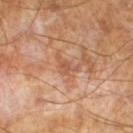{
  "biopsy_status": "not biopsied; imaged during a skin examination",
  "patient": {
    "sex": "male",
    "age_approx": 65
  },
  "image": {
    "source": "total-body photography crop",
    "field_of_view_mm": 15
  }
}